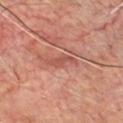The lesion was tiled from a total-body skin photograph and was not biopsied.
A male patient aged 58–62.
The lesion is located on the front of the torso.
A region of skin cropped from a whole-body photographic capture, roughly 15 mm wide.